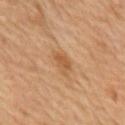Part of a total-body skin-imaging series; this lesion was reviewed on a skin check and was not flagged for biopsy.